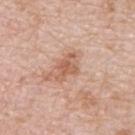This is a white-light tile. A female patient in their mid-40s. Automated tile analysis of the lesion measured an average lesion color of about L≈59 a*≈22 b*≈31 (CIELAB) and a normalized lesion–skin contrast near 7. The analysis additionally found border irregularity of about 3 on a 0–10 scale, internal color variation of about 3.5 on a 0–10 scale, and radial color variation of about 1. And it measured a nevus-likeness score of about 0/100 and a detector confidence of about 100 out of 100 that the crop contains a lesion. About 4 mm across. From the upper back. A roughly 15 mm field-of-view crop from a total-body skin photograph.